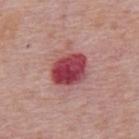Q: Is there a histopathology result?
A: imaged on a skin check; not biopsied
Q: What is the imaging modality?
A: total-body-photography crop, ~15 mm field of view
Q: What are the patient's age and sex?
A: male, aged 73 to 77
Q: What is the lesion's diameter?
A: ~4.5 mm (longest diameter)
Q: Illumination type?
A: white-light illumination
Q: What is the anatomic site?
A: the mid back